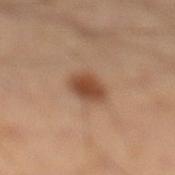notes: no biopsy performed (imaged during a skin exam); anatomic site: the left leg; patient: male, about 50 years old; imaging modality: ~15 mm crop, total-body skin-cancer survey; size: about 3.5 mm; image-analysis metrics: border irregularity of about 1.5 on a 0–10 scale, a within-lesion color-variation index near 2.5/10, and radial color variation of about 0.5; lighting: cross-polarized illumination.